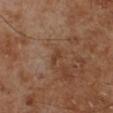  biopsy_status: not biopsied; imaged during a skin examination
  lesion_size:
    long_diameter_mm_approx: 2.5
  automated_metrics:
    cielab_L: 38
    cielab_a: 19
    cielab_b: 29
  site: left lower leg
  lighting: cross-polarized
  image:
    source: total-body photography crop
    field_of_view_mm: 15
  patient:
    sex: male
    age_approx: 70